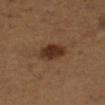Acquisition and patient details: A 15 mm crop from a total-body photograph taken for skin-cancer surveillance. Longest diameter approximately 3.5 mm. A female patient in their mid- to late 50s. Located on the leg. Captured under cross-polarized illumination.